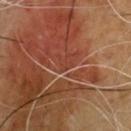Impression: Imaged during a routine full-body skin examination; the lesion was not biopsied and no histopathology is available. Acquisition and patient details: A male patient, about 65 years old. Located on the chest. This is a cross-polarized tile. A 15 mm close-up extracted from a 3D total-body photography capture.